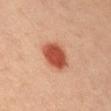Assessment: Captured during whole-body skin photography for melanoma surveillance; the lesion was not biopsied. Acquisition and patient details: The tile uses cross-polarized illumination. From the right upper arm. A 15 mm crop from a total-body photograph taken for skin-cancer surveillance. A female subject aged around 45. Measured at roughly 4 mm in maximum diameter.